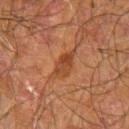The lesion was tiled from a total-body skin photograph and was not biopsied. Imaged with cross-polarized lighting. From the arm. A male patient, aged approximately 70. This image is a 15 mm lesion crop taken from a total-body photograph.